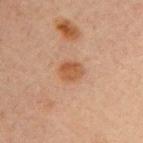| feature | finding |
|---|---|
| workup | catalogued during a skin exam; not biopsied |
| automated lesion analysis | a lesion color around L≈45 a*≈20 b*≈31 in CIELAB; a border-irregularity rating of about 2/10 and a peripheral color-asymmetry measure near 1; a nevus-likeness score of about 80/100 and a lesion-detection confidence of about 100/100 |
| patient | male, aged approximately 30 |
| location | the right upper arm |
| image source | total-body-photography crop, ~15 mm field of view |